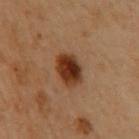workup = no biopsy performed (imaged during a skin exam) | patient = female, in their 60s | anatomic site = the upper back | image = ~15 mm crop, total-body skin-cancer survey | lesion diameter = ≈3.5 mm | tile lighting = cross-polarized | automated lesion analysis = an average lesion color of about L≈30 a*≈20 b*≈29 (CIELAB); a border-irregularity rating of about 1.5/10, internal color variation of about 6 on a 0–10 scale, and radial color variation of about 2.